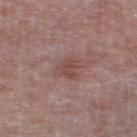No biopsy was performed on this lesion — it was imaged during a full skin examination and was not determined to be concerning.
A roughly 15 mm field-of-view crop from a total-body skin photograph.
An algorithmic analysis of the crop reported border irregularity of about 4 on a 0–10 scale, a color-variation rating of about 1.5/10, and peripheral color asymmetry of about 0.5. And it measured an automated nevus-likeness rating near 0 out of 100 and a lesion-detection confidence of about 100/100.
A male patient, aged 63–67.
From the left thigh.